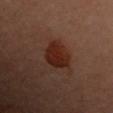Impression:
Recorded during total-body skin imaging; not selected for excision or biopsy.
Background:
This is a cross-polarized tile. Automated image analysis of the tile measured a lesion color around L≈20 a*≈20 b*≈23 in CIELAB, about 8 CIELAB-L* units darker than the surrounding skin, and a lesion-to-skin contrast of about 10.5 (normalized; higher = more distinct). The analysis additionally found a nevus-likeness score of about 95/100 and lesion-presence confidence of about 100/100. A roughly 15 mm field-of-view crop from a total-body skin photograph. A female subject, aged around 60. The lesion is on the left arm.